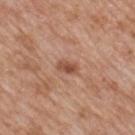Clinical impression: This lesion was catalogued during total-body skin photography and was not selected for biopsy. Image and clinical context: A lesion tile, about 15 mm wide, cut from a 3D total-body photograph. Located on the mid back. A male patient approximately 60 years of age.